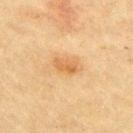Impression:
Recorded during total-body skin imaging; not selected for excision or biopsy.
Image and clinical context:
About 3 mm across. A region of skin cropped from a whole-body photographic capture, roughly 15 mm wide. Automated tile analysis of the lesion measured a mean CIELAB color near L≈57 a*≈20 b*≈40 and roughly 8 lightness units darker than nearby skin. And it measured an automated nevus-likeness rating near 65 out of 100 and a lesion-detection confidence of about 100/100. The lesion is located on the right upper arm. A female subject, about 55 years old.